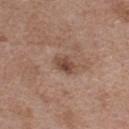<record>
  <biopsy_status>not biopsied; imaged during a skin examination</biopsy_status>
  <site>back</site>
  <lighting>white-light</lighting>
  <lesion_size>
    <long_diameter_mm_approx>3.0</long_diameter_mm_approx>
  </lesion_size>
  <image>
    <source>total-body photography crop</source>
    <field_of_view_mm>15</field_of_view_mm>
  </image>
  <patient>
    <sex>female</sex>
    <age_approx>40</age_approx>
  </patient>
</record>